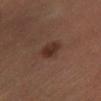Recorded during total-body skin imaging; not selected for excision or biopsy.
A male subject aged around 50.
Longest diameter approximately 2.5 mm.
Imaged with cross-polarized lighting.
From the left lower leg.
A close-up tile cropped from a whole-body skin photograph, about 15 mm across.
The lesion-visualizer software estimated a classifier nevus-likeness of about 95/100 and a lesion-detection confidence of about 100/100.